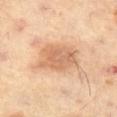Q: Was a biopsy performed?
A: catalogued during a skin exam; not biopsied
Q: Lesion location?
A: the left thigh
Q: What kind of image is this?
A: total-body-photography crop, ~15 mm field of view
Q: What are the patient's age and sex?
A: male, approximately 60 years of age